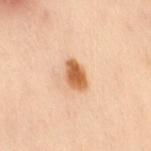Case summary:
– notes · imaged on a skin check; not biopsied
– image · 15 mm crop, total-body photography
– body site · the leg
– patient · female, about 50 years old